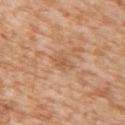Recorded during total-body skin imaging; not selected for excision or biopsy.